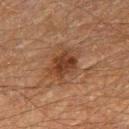Part of a total-body skin-imaging series; this lesion was reviewed on a skin check and was not flagged for biopsy.
Located on the right thigh.
Captured under cross-polarized illumination.
Longest diameter approximately 4.5 mm.
The subject is a male aged around 60.
A 15 mm crop from a total-body photograph taken for skin-cancer surveillance.